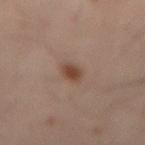workup = total-body-photography surveillance lesion; no biopsy | lesion size = about 2.5 mm | patient = male, aged around 50 | tile lighting = cross-polarized illumination | body site = the lower back | imaging modality = ~15 mm tile from a whole-body skin photo.